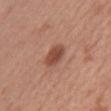Q: Was a biopsy performed?
A: imaged on a skin check; not biopsied
Q: What kind of image is this?
A: 15 mm crop, total-body photography
Q: How large is the lesion?
A: ~3.5 mm (longest diameter)
Q: Illumination type?
A: white-light illumination
Q: What is the anatomic site?
A: the abdomen
Q: Patient demographics?
A: male, aged approximately 65